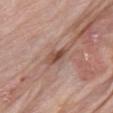Case summary:
• follow-up · catalogued during a skin exam; not biopsied
• location · the left upper arm
• acquisition · ~15 mm crop, total-body skin-cancer survey
• illumination · white-light illumination
• patient · male, aged 78–82
• diameter · about 3 mm